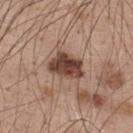Captured during whole-body skin photography for melanoma surveillance; the lesion was not biopsied. On the back. Approximately 4.5 mm at its widest. This image is a 15 mm lesion crop taken from a total-body photograph. A male subject, aged approximately 50. Automated image analysis of the tile measured an area of roughly 10 mm² and an eccentricity of roughly 0.75. And it measured border irregularity of about 2.5 on a 0–10 scale, internal color variation of about 5 on a 0–10 scale, and radial color variation of about 1.5. The software also gave an automated nevus-likeness rating near 75 out of 100 and a lesion-detection confidence of about 100/100. The tile uses white-light illumination.